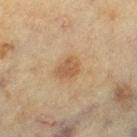• image source: total-body-photography crop, ~15 mm field of view
• lesion diameter: about 3 mm
• patient: female, about 55 years old
• anatomic site: the left thigh
• lighting: cross-polarized illumination